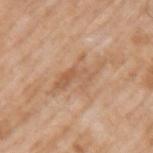Assessment:
Captured during whole-body skin photography for melanoma surveillance; the lesion was not biopsied.
Image and clinical context:
On the left upper arm. A male patient aged 58–62. A lesion tile, about 15 mm wide, cut from a 3D total-body photograph.